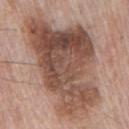Imaged during a routine full-body skin examination; the lesion was not biopsied and no histopathology is available. The lesion is on the chest. The tile uses white-light illumination. A lesion tile, about 15 mm wide, cut from a 3D total-body photograph. The lesion's longest dimension is about 13 mm. The total-body-photography lesion software estimated a lesion color around L≈50 a*≈19 b*≈26 in CIELAB, roughly 15 lightness units darker than nearby skin, and a lesion-to-skin contrast of about 10 (normalized; higher = more distinct). It also reported border irregularity of about 5 on a 0–10 scale, a color-variation rating of about 8/10, and a peripheral color-asymmetry measure near 2.5. It also reported a lesion-detection confidence of about 100/100. A male subject, roughly 70 years of age.